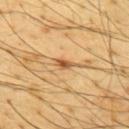Q: Was a biopsy performed?
A: catalogued during a skin exam; not biopsied
Q: What is the anatomic site?
A: the upper back
Q: What did automated image analysis measure?
A: a footprint of about 2.5 mm² and an outline eccentricity of about 0.8 (0 = round, 1 = elongated); a border-irregularity index near 2.5/10, a color-variation rating of about 3/10, and radial color variation of about 1
Q: Lesion size?
A: about 2 mm
Q: What are the patient's age and sex?
A: male, aged 63–67
Q: What kind of image is this?
A: ~15 mm tile from a whole-body skin photo
Q: Illumination type?
A: cross-polarized